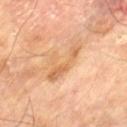* follow-up — imaged on a skin check; not biopsied
* body site — the left thigh
* subject — male, aged around 70
* image — total-body-photography crop, ~15 mm field of view